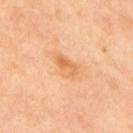Assessment: Captured during whole-body skin photography for melanoma surveillance; the lesion was not biopsied.